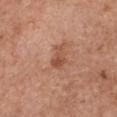follow-up: total-body-photography surveillance lesion; no biopsy
acquisition: 15 mm crop, total-body photography
site: the front of the torso
subject: female, in their mid- to late 50s
lesion size: ~3 mm (longest diameter)
illumination: white-light